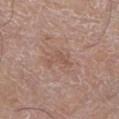Case summary:
- workup · no biopsy performed (imaged during a skin exam)
- lighting · white-light
- subject · male, in their 60s
- imaging modality · ~15 mm tile from a whole-body skin photo
- diameter · about 3.5 mm
- location · the left lower leg